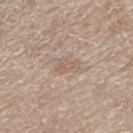Background:
Cropped from a whole-body photographic skin survey; the tile spans about 15 mm. On the left thigh. A male patient, aged 78–82. The recorded lesion diameter is about 2.5 mm. Captured under white-light illumination.